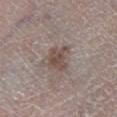biopsy_status: not biopsied; imaged during a skin examination
image:
  source: total-body photography crop
  field_of_view_mm: 15
patient:
  sex: male
  age_approx: 85+
site: leg